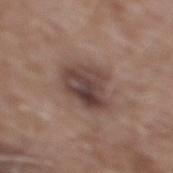Clinical impression:
Captured during whole-body skin photography for melanoma surveillance; the lesion was not biopsied.
Background:
A 15 mm close-up tile from a total-body photography series done for melanoma screening. This is a white-light tile. A male patient, about 60 years old. An algorithmic analysis of the crop reported a lesion area of about 15 mm² and a shape-asymmetry score of about 0.25 (0 = symmetric). The software also gave roughly 11 lightness units darker than nearby skin and a normalized border contrast of about 9. About 5 mm across. From the mid back.